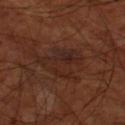Q: Is there a histopathology result?
A: total-body-photography surveillance lesion; no biopsy
Q: Where on the body is the lesion?
A: the left thigh
Q: What is the lesion's diameter?
A: about 6 mm
Q: How was this image acquired?
A: 15 mm crop, total-body photography
Q: What are the patient's age and sex?
A: male, aged approximately 70
Q: Automated lesion metrics?
A: an average lesion color of about L≈23 a*≈18 b*≈22 (CIELAB), about 5 CIELAB-L* units darker than the surrounding skin, and a lesion-to-skin contrast of about 6.5 (normalized; higher = more distinct); border irregularity of about 7 on a 0–10 scale, internal color variation of about 4.5 on a 0–10 scale, and radial color variation of about 1.5